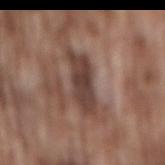Clinical summary: Located on the mid back. A roughly 15 mm field-of-view crop from a total-body skin photograph. Approximately 6 mm at its widest. A male patient, in their mid- to late 70s.